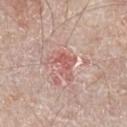No biopsy was performed on this lesion — it was imaged during a full skin examination and was not determined to be concerning. Imaged with white-light lighting. Automated image analysis of the tile measured a lesion area of about 7.5 mm² and a symmetry-axis asymmetry near 0.55. The analysis additionally found a mean CIELAB color near L≈59 a*≈25 b*≈24, roughly 8 lightness units darker than nearby skin, and a normalized lesion–skin contrast near 5.5. The analysis additionally found an automated nevus-likeness rating near 0 out of 100. The patient is a male aged 63–67. Measured at roughly 4 mm in maximum diameter. A roughly 15 mm field-of-view crop from a total-body skin photograph. The lesion is on the chest.